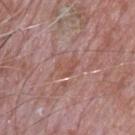<case>
<biopsy_status>not biopsied; imaged during a skin examination</biopsy_status>
<site>back</site>
<image>
  <source>total-body photography crop</source>
  <field_of_view_mm>15</field_of_view_mm>
</image>
<lighting>white-light</lighting>
<patient>
  <sex>male</sex>
  <age_approx>65</age_approx>
</patient>
<automated_metrics>
  <area_mm2_approx>4.0</area_mm2_approx>
  <eccentricity>0.75</eccentricity>
  <shape_asymmetry>0.45</shape_asymmetry>
  <cielab_L>52</cielab_L>
  <cielab_a>22</cielab_a>
  <cielab_b>26</cielab_b>
  <nevus_likeness_0_100>0</nevus_likeness_0_100>
  <lesion_detection_confidence_0_100>80</lesion_detection_confidence_0_100>
</automated_metrics>
<lesion_size>
  <long_diameter_mm_approx>3.0</long_diameter_mm_approx>
</lesion_size>
</case>